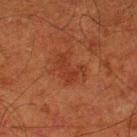Assessment:
No biopsy was performed on this lesion — it was imaged during a full skin examination and was not determined to be concerning.
Context:
Captured under cross-polarized illumination. Located on the left lower leg. Approximately 4 mm at its widest. Cropped from a total-body skin-imaging series; the visible field is about 15 mm. A male patient, aged approximately 80.